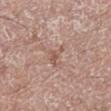The lesion was photographed on a routine skin check and not biopsied; there is no pathology result.
A 15 mm close-up extracted from a 3D total-body photography capture.
The lesion is located on the right lower leg.
Approximately 3 mm at its widest.
A male subject aged approximately 60.
Automated image analysis of the tile measured a shape eccentricity near 0.9 and two-axis asymmetry of about 0.55. The software also gave a mean CIELAB color near L≈55 a*≈20 b*≈26 and a normalized border contrast of about 5.5.